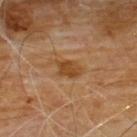Notes:
– biopsy status: imaged on a skin check; not biopsied
– patient: male, in their 60s
– automated lesion analysis: an eccentricity of roughly 0.85
– acquisition: 15 mm crop, total-body photography
– site: the front of the torso
– lighting: cross-polarized illumination